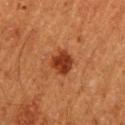Captured during whole-body skin photography for melanoma surveillance; the lesion was not biopsied. The tile uses cross-polarized illumination. The lesion is located on the left upper arm. A 15 mm close-up extracted from a 3D total-body photography capture. The lesion-visualizer software estimated a footprint of about 7.5 mm², a shape eccentricity near 0.45, and a symmetry-axis asymmetry near 0.15. The software also gave border irregularity of about 1.5 on a 0–10 scale and internal color variation of about 3 on a 0–10 scale. And it measured lesion-presence confidence of about 100/100. A male subject, aged 58–62.